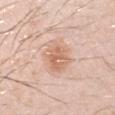This lesion was catalogued during total-body skin photography and was not selected for biopsy.
Measured at roughly 4.5 mm in maximum diameter.
This is a white-light tile.
The subject is a male aged 28–32.
From the left upper arm.
This image is a 15 mm lesion crop taken from a total-body photograph.